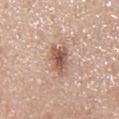– workup — total-body-photography surveillance lesion; no biopsy
– illumination — white-light
– size — ~4.5 mm (longest diameter)
– TBP lesion metrics — a lesion color around L≈56 a*≈20 b*≈27 in CIELAB, roughly 13 lightness units darker than nearby skin, and a normalized border contrast of about 8.5; a border-irregularity rating of about 3.5/10 and internal color variation of about 4.5 on a 0–10 scale; an automated nevus-likeness rating near 90 out of 100 and a lesion-detection confidence of about 100/100
– acquisition — ~15 mm crop, total-body skin-cancer survey
– patient — female, roughly 50 years of age
– site — the mid back